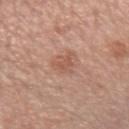| key | value |
|---|---|
| follow-up | total-body-photography surveillance lesion; no biopsy |
| imaging modality | 15 mm crop, total-body photography |
| patient | female, aged approximately 50 |
| lighting | white-light |
| anatomic site | the right forearm |